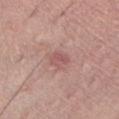Imaged during a routine full-body skin examination; the lesion was not biopsied and no histopathology is available.
An algorithmic analysis of the crop reported a footprint of about 4 mm² and an eccentricity of roughly 0.65. And it measured a lesion color around L≈54 a*≈24 b*≈21 in CIELAB, about 8 CIELAB-L* units darker than the surrounding skin, and a normalized border contrast of about 5.5. It also reported a nevus-likeness score of about 0/100.
A female subject aged approximately 65.
Captured under white-light illumination.
On the front of the torso.
A close-up tile cropped from a whole-body skin photograph, about 15 mm across.